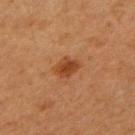Image and clinical context: A 15 mm close-up extracted from a 3D total-body photography capture. The lesion-visualizer software estimated an average lesion color of about L≈37 a*≈23 b*≈33 (CIELAB), roughly 9 lightness units darker than nearby skin, and a normalized border contrast of about 8.5. The subject is a female approximately 40 years of age. This is a cross-polarized tile. The recorded lesion diameter is about 2.5 mm. The lesion is located on the right upper arm.